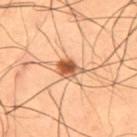Findings:
• biopsy status · catalogued during a skin exam; not biopsied
• illumination · cross-polarized illumination
• anatomic site · the right thigh
• acquisition · ~15 mm tile from a whole-body skin photo
• patient · male, aged around 50
• automated lesion analysis · a mean CIELAB color near L≈45 a*≈21 b*≈31, about 14 CIELAB-L* units darker than the surrounding skin, and a normalized lesion–skin contrast near 10.5; a border-irregularity rating of about 2/10, a within-lesion color-variation index near 5/10, and a peripheral color-asymmetry measure near 1.5
• lesion diameter · ~3 mm (longest diameter)